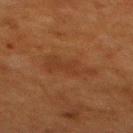Context:
Captured under cross-polarized illumination. From the back. A 15 mm crop from a total-body photograph taken for skin-cancer surveillance. The total-body-photography lesion software estimated an eccentricity of roughly 0.9 and a symmetry-axis asymmetry near 0.4. And it measured an average lesion color of about L≈30 a*≈20 b*≈29 (CIELAB), about 4 CIELAB-L* units darker than the surrounding skin, and a lesion-to-skin contrast of about 4.5 (normalized; higher = more distinct). The software also gave a detector confidence of about 100 out of 100 that the crop contains a lesion. Longest diameter approximately 5 mm. A female patient, about 50 years old.